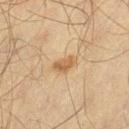The lesion was tiled from a total-body skin photograph and was not biopsied.
The patient is a male roughly 50 years of age.
The lesion is on the leg.
A roughly 15 mm field-of-view crop from a total-body skin photograph.
Approximately 3 mm at its widest.
Captured under cross-polarized illumination.